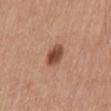Imaged during a routine full-body skin examination; the lesion was not biopsied and no histopathology is available.
An algorithmic analysis of the crop reported a lesion color around L≈48 a*≈24 b*≈30 in CIELAB, roughly 14 lightness units darker than nearby skin, and a normalized border contrast of about 10. And it measured an automated nevus-likeness rating near 95 out of 100 and a detector confidence of about 100 out of 100 that the crop contains a lesion.
A male patient in their mid-50s.
Captured under white-light illumination.
On the mid back.
A close-up tile cropped from a whole-body skin photograph, about 15 mm across.